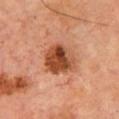Impression:
Imaged during a routine full-body skin examination; the lesion was not biopsied and no histopathology is available.
Acquisition and patient details:
The lesion-visualizer software estimated a border-irregularity rating of about 2/10 and a color-variation rating of about 9/10. Imaged with cross-polarized lighting. A 15 mm crop from a total-body photograph taken for skin-cancer surveillance. The subject is a male approximately 70 years of age. Located on the chest.